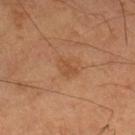{"biopsy_status": "not biopsied; imaged during a skin examination", "lesion_size": {"long_diameter_mm_approx": 2.5}, "automated_metrics": {"area_mm2_approx": 3.5, "eccentricity": 0.7, "cielab_L": 49, "cielab_a": 22, "cielab_b": 35, "vs_skin_darker_L": 6.0, "vs_skin_contrast_norm": 5.0}, "patient": {"sex": "male", "age_approx": 60}, "lighting": "cross-polarized", "site": "right lower leg", "image": {"source": "total-body photography crop", "field_of_view_mm": 15}}